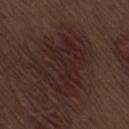Q: Is there a histopathology result?
A: imaged on a skin check; not biopsied
Q: What is the anatomic site?
A: the leg
Q: Who is the patient?
A: male, about 70 years old
Q: Illumination type?
A: white-light
Q: What is the lesion's diameter?
A: ~7.5 mm (longest diameter)
Q: What is the imaging modality?
A: ~15 mm crop, total-body skin-cancer survey